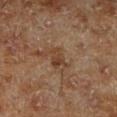follow-up — total-body-photography surveillance lesion; no biopsy | site — the leg | image — ~15 mm tile from a whole-body skin photo | subject — male, aged approximately 70 | lesion size — ≈3 mm | TBP lesion metrics — a mean CIELAB color near L≈39 a*≈18 b*≈29 and a normalized lesion–skin contrast near 7; border irregularity of about 4 on a 0–10 scale, internal color variation of about 2 on a 0–10 scale, and radial color variation of about 0.5 | lighting — cross-polarized.